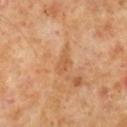  biopsy_status: not biopsied; imaged during a skin examination
  lesion_size:
    long_diameter_mm_approx: 3.0
  automated_metrics:
    area_mm2_approx: 3.5
    eccentricity: 0.75
    shape_asymmetry: 0.3
    border_irregularity_0_10: 3.0
    color_variation_0_10: 1.5
    peripheral_color_asymmetry: 0.5
  image:
    source: total-body photography crop
    field_of_view_mm: 15
  site: right lower leg
  patient:
    sex: male
    age_approx: 60
  lighting: cross-polarized